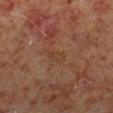A 15 mm close-up tile from a total-body photography series done for melanoma screening. A male subject, roughly 60 years of age. An algorithmic analysis of the crop reported internal color variation of about 0.5 on a 0–10 scale and a peripheral color-asymmetry measure near 0. And it measured a classifier nevus-likeness of about 0/100 and lesion-presence confidence of about 100/100. Measured at roughly 2.5 mm in maximum diameter. The tile uses cross-polarized illumination. From the right lower leg.